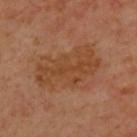| feature | finding |
|---|---|
| notes | no biopsy performed (imaged during a skin exam) |
| patient | male, aged around 45 |
| TBP lesion metrics | a border-irregularity rating of about 5/10, a color-variation rating of about 3/10, and radial color variation of about 1; an automated nevus-likeness rating near 0 out of 100 and lesion-presence confidence of about 100/100 |
| diameter | about 7.5 mm |
| lighting | cross-polarized |
| anatomic site | the upper back |
| image | ~15 mm crop, total-body skin-cancer survey |